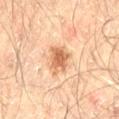Clinical impression: No biopsy was performed on this lesion — it was imaged during a full skin examination and was not determined to be concerning. Image and clinical context: A male subject aged 63–67. Imaged with cross-polarized lighting. A roughly 15 mm field-of-view crop from a total-body skin photograph. From the left thigh.